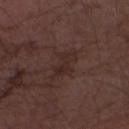Clinical impression: Captured during whole-body skin photography for melanoma surveillance; the lesion was not biopsied. Acquisition and patient details: The patient is a male aged approximately 75. On the right forearm. A lesion tile, about 15 mm wide, cut from a 3D total-body photograph. Automated tile analysis of the lesion measured a lesion area of about 6 mm² and a symmetry-axis asymmetry near 0.45. About 4 mm across. The tile uses white-light illumination.